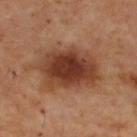{
  "biopsy_status": "not biopsied; imaged during a skin examination",
  "patient": {
    "sex": "male",
    "age_approx": 55
  },
  "image": {
    "source": "total-body photography crop",
    "field_of_view_mm": 15
  },
  "site": "back"
}